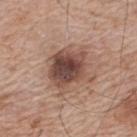Assessment:
This lesion was catalogued during total-body skin photography and was not selected for biopsy.
Acquisition and patient details:
This is a white-light tile. Cropped from a whole-body photographic skin survey; the tile spans about 15 mm. The subject is a male roughly 65 years of age. The lesion-visualizer software estimated an eccentricity of roughly 0.45 and a symmetry-axis asymmetry near 0.25. It also reported an average lesion color of about L≈47 a*≈19 b*≈25 (CIELAB). The analysis additionally found a border-irregularity index near 3.5/10 and internal color variation of about 7.5 on a 0–10 scale. And it measured an automated nevus-likeness rating near 20 out of 100 and a detector confidence of about 100 out of 100 that the crop contains a lesion. The recorded lesion diameter is about 5 mm. The lesion is located on the upper back.